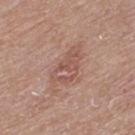No biopsy was performed on this lesion — it was imaged during a full skin examination and was not determined to be concerning. Measured at roughly 5 mm in maximum diameter. A male subject in their mid- to late 70s. Imaged with white-light lighting. The lesion is on the upper back. Cropped from a whole-body photographic skin survey; the tile spans about 15 mm.